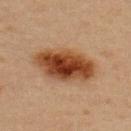The lesion was photographed on a routine skin check and not biopsied; there is no pathology result. The subject is a female in their mid- to late 40s. Measured at roughly 7.5 mm in maximum diameter. A 15 mm crop from a total-body photograph taken for skin-cancer surveillance. The lesion is on the back. This is a cross-polarized tile. Automated image analysis of the tile measured an average lesion color of about L≈38 a*≈21 b*≈32 (CIELAB), a lesion–skin lightness drop of about 15, and a lesion-to-skin contrast of about 12 (normalized; higher = more distinct). And it measured a border-irregularity index near 1.5/10 and a within-lesion color-variation index near 8/10.